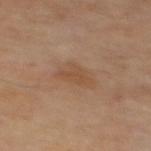{"biopsy_status": "not biopsied; imaged during a skin examination", "image": {"source": "total-body photography crop", "field_of_view_mm": 15}, "site": "mid back"}